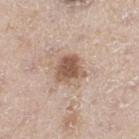| feature | finding |
|---|---|
| workup | catalogued during a skin exam; not biopsied |
| tile lighting | white-light illumination |
| location | the left thigh |
| lesion diameter | about 3.5 mm |
| subject | male, approximately 65 years of age |
| image | total-body-photography crop, ~15 mm field of view |
| image-analysis metrics | an eccentricity of roughly 0.5 and two-axis asymmetry of about 0.35; an average lesion color of about L≈55 a*≈18 b*≈28 (CIELAB), about 13 CIELAB-L* units darker than the surrounding skin, and a normalized lesion–skin contrast near 9 |